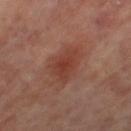notes: catalogued during a skin exam; not biopsied | tile lighting: cross-polarized | automated metrics: a lesion area of about 11 mm², an outline eccentricity of about 0.75 (0 = round, 1 = elongated), and two-axis asymmetry of about 0.25; a lesion color around L≈39 a*≈24 b*≈27 in CIELAB; an automated nevus-likeness rating near 65 out of 100 and lesion-presence confidence of about 100/100 | imaging modality: total-body-photography crop, ~15 mm field of view | patient: female, approximately 65 years of age | lesion diameter: about 4.5 mm | anatomic site: the leg.